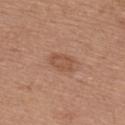The lesion was photographed on a routine skin check and not biopsied; there is no pathology result.
A roughly 15 mm field-of-view crop from a total-body skin photograph.
A female patient in their mid- to late 30s.
The lesion is on the upper back.
Captured under white-light illumination.